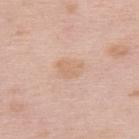Clinical impression: The lesion was tiled from a total-body skin photograph and was not biopsied. Acquisition and patient details: Captured under white-light illumination. About 3 mm across. Cropped from a total-body skin-imaging series; the visible field is about 15 mm. A female subject, in their mid- to late 60s. On the upper back. The total-body-photography lesion software estimated an area of roughly 4 mm² and two-axis asymmetry of about 0.35. And it measured an automated nevus-likeness rating near 0 out of 100.